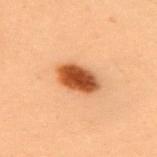Clinical impression:
Captured during whole-body skin photography for melanoma surveillance; the lesion was not biopsied.
Background:
The lesion is located on the upper back. Approximately 4.5 mm at its widest. A roughly 15 mm field-of-view crop from a total-body skin photograph. Automated image analysis of the tile measured an eccentricity of roughly 0.8 and two-axis asymmetry of about 0.15. The software also gave border irregularity of about 1.5 on a 0–10 scale and a within-lesion color-variation index near 5.5/10. A female subject aged 38–42. Captured under cross-polarized illumination.